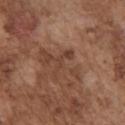follow-up: total-body-photography surveillance lesion; no biopsy
TBP lesion metrics: a lesion area of about 4 mm², an eccentricity of roughly 0.95, and a shape-asymmetry score of about 0.65 (0 = symmetric); a border-irregularity rating of about 8/10, a color-variation rating of about 0/10, and a peripheral color-asymmetry measure near 0
anatomic site: the chest
tile lighting: white-light
patient: male, approximately 75 years of age
size: ≈4 mm
acquisition: 15 mm crop, total-body photography